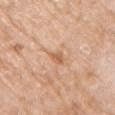Findings:
• follow-up: no biopsy performed (imaged during a skin exam)
• site: the chest
• acquisition: ~15 mm tile from a whole-body skin photo
• patient: female, aged around 75